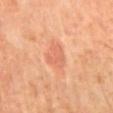The lesion was photographed on a routine skin check and not biopsied; there is no pathology result. A roughly 15 mm field-of-view crop from a total-body skin photograph. A male patient aged 58 to 62. Located on the mid back. This is a cross-polarized tile.